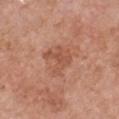Acquisition and patient details: A female subject aged around 60. Automated image analysis of the tile measured a footprint of about 7 mm² and an eccentricity of roughly 0.1. The analysis additionally found a mean CIELAB color near L≈53 a*≈25 b*≈32, about 8 CIELAB-L* units darker than the surrounding skin, and a normalized border contrast of about 5.5. From the front of the torso. A 15 mm close-up tile from a total-body photography series done for melanoma screening. This is a white-light tile. About 3.5 mm across.